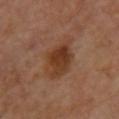  biopsy_status: not biopsied; imaged during a skin examination
  patient:
    sex: female
    age_approx: 65
  site: front of the torso
  image:
    source: total-body photography crop
    field_of_view_mm: 15